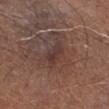Clinical impression:
Imaged during a routine full-body skin examination; the lesion was not biopsied and no histopathology is available.
Acquisition and patient details:
The lesion is located on the right lower leg. Approximately 3 mm at its widest. A male patient aged around 65. This image is a 15 mm lesion crop taken from a total-body photograph.